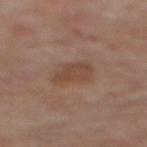Findings:
- notes: no biopsy performed (imaged during a skin exam)
- illumination: cross-polarized illumination
- site: the mid back
- imaging modality: ~15 mm tile from a whole-body skin photo
- automated lesion analysis: a lesion color around L≈42 a*≈17 b*≈26 in CIELAB and a lesion-to-skin contrast of about 6.5 (normalized; higher = more distinct); a nevus-likeness score of about 0/100 and a detector confidence of about 100 out of 100 that the crop contains a lesion
- subject: male, aged 68–72
- lesion size: about 3.5 mm